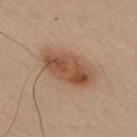{
  "biopsy_status": "not biopsied; imaged during a skin examination",
  "patient": {
    "sex": "male",
    "age_approx": 50
  },
  "lesion_size": {
    "long_diameter_mm_approx": 6.0
  },
  "site": "left arm",
  "image": {
    "source": "total-body photography crop",
    "field_of_view_mm": 15
  },
  "automated_metrics": {
    "area_mm2_approx": 16.0,
    "eccentricity": 0.8,
    "shape_asymmetry": 0.15,
    "vs_skin_darker_L": 9.0,
    "vs_skin_contrast_norm": 8.0,
    "border_irregularity_0_10": 2.0,
    "color_variation_0_10": 4.5,
    "peripheral_color_asymmetry": 1.5,
    "nevus_likeness_0_100": 95,
    "lesion_detection_confidence_0_100": 100
  }
}